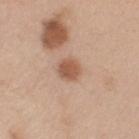Clinical impression:
Recorded during total-body skin imaging; not selected for excision or biopsy.
Background:
A male patient, roughly 70 years of age. From the left upper arm. A region of skin cropped from a whole-body photographic capture, roughly 15 mm wide.